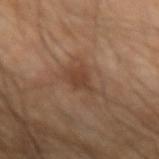Assessment:
Imaged during a routine full-body skin examination; the lesion was not biopsied and no histopathology is available.
Acquisition and patient details:
This is a cross-polarized tile. A 15 mm close-up extracted from a 3D total-body photography capture. Located on the arm. A male patient, in their 70s.